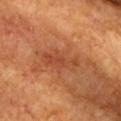workup: imaged on a skin check; not biopsied
image source: ~15 mm tile from a whole-body skin photo
body site: the chest
patient: female, approximately 75 years of age
image-analysis metrics: a mean CIELAB color near L≈48 a*≈29 b*≈38, a lesion–skin lightness drop of about 8, and a lesion-to-skin contrast of about 5.5 (normalized; higher = more distinct); border irregularity of about 4 on a 0–10 scale, internal color variation of about 3.5 on a 0–10 scale, and peripheral color asymmetry of about 1; a classifier nevus-likeness of about 5/100
tile lighting: cross-polarized illumination
lesion size: ≈5 mm